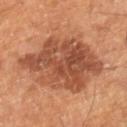Captured during whole-body skin photography for melanoma surveillance; the lesion was not biopsied. Captured under cross-polarized illumination. A male patient aged 58–62. Approximately 10 mm at its widest. The total-body-photography lesion software estimated a lesion area of about 43 mm², a shape eccentricity near 0.75, and a shape-asymmetry score of about 0.2 (0 = symmetric). And it measured an average lesion color of about L≈48 a*≈25 b*≈32 (CIELAB) and a lesion–skin lightness drop of about 12. And it measured a border-irregularity index near 3.5/10, a within-lesion color-variation index near 5.5/10, and peripheral color asymmetry of about 2. And it measured a nevus-likeness score of about 55/100 and lesion-presence confidence of about 100/100. Located on the right lower leg. Cropped from a whole-body photographic skin survey; the tile spans about 15 mm.